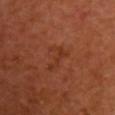Imaged during a routine full-body skin examination; the lesion was not biopsied and no histopathology is available. Imaged with cross-polarized lighting. Located on the chest. A roughly 15 mm field-of-view crop from a total-body skin photograph. The recorded lesion diameter is about 3.5 mm. A female subject, aged 53–57.